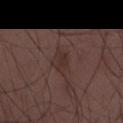biopsy status: no biopsy performed (imaged during a skin exam)
lesion size: about 3.5 mm
image-analysis metrics: an area of roughly 6 mm², an outline eccentricity of about 0.65 (0 = round, 1 = elongated), and a symmetry-axis asymmetry near 0.25; roughly 5 lightness units darker than nearby skin and a lesion-to-skin contrast of about 5.5 (normalized; higher = more distinct); a detector confidence of about 100 out of 100 that the crop contains a lesion
lighting: white-light
patient: male, aged approximately 30
imaging modality: ~15 mm crop, total-body skin-cancer survey
anatomic site: the lower back